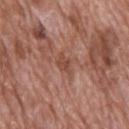Case summary:
• notes — imaged on a skin check; not biopsied
• site — the upper back
• automated metrics — an average lesion color of about L≈47 a*≈23 b*≈28 (CIELAB), about 8 CIELAB-L* units darker than the surrounding skin, and a normalized border contrast of about 6; a classifier nevus-likeness of about 0/100
• size — ~2.5 mm (longest diameter)
• acquisition — 15 mm crop, total-body photography
• illumination — white-light
• patient — male, aged 68–72